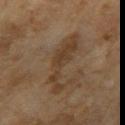biopsy status=catalogued during a skin exam; not biopsied
acquisition=~15 mm crop, total-body skin-cancer survey
subject=female, roughly 60 years of age
lighting=cross-polarized
size=≈7 mm
body site=the arm